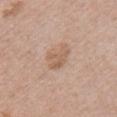| key | value |
|---|---|
| biopsy status | imaged on a skin check; not biopsied |
| subject | female, aged 38 to 42 |
| image source | ~15 mm tile from a whole-body skin photo |
| anatomic site | the front of the torso |